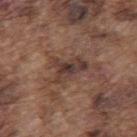This lesion was catalogued during total-body skin photography and was not selected for biopsy. The lesion-visualizer software estimated a mean CIELAB color near L≈37 a*≈17 b*≈22, a lesion–skin lightness drop of about 9, and a normalized border contrast of about 8.5. On the mid back. The subject is a male roughly 75 years of age. Cropped from a total-body skin-imaging series; the visible field is about 15 mm. The lesion's longest dimension is about 4.5 mm. Captured under white-light illumination.